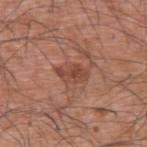follow-up — total-body-photography surveillance lesion; no biopsy | lighting — white-light illumination | site — the upper back | size — ~3.5 mm (longest diameter) | imaging modality — ~15 mm tile from a whole-body skin photo | subject — male, aged 58 to 62.